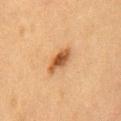{"biopsy_status": "not biopsied; imaged during a skin examination", "lesion_size": {"long_diameter_mm_approx": 4.0}, "site": "front of the torso", "automated_metrics": {"area_mm2_approx": 5.5, "eccentricity": 0.9, "shape_asymmetry": 0.2, "border_irregularity_0_10": 2.5, "color_variation_0_10": 4.5}, "patient": {"sex": "female", "age_approx": 60}, "image": {"source": "total-body photography crop", "field_of_view_mm": 15}, "lighting": "cross-polarized"}